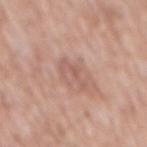No biopsy was performed on this lesion — it was imaged during a full skin examination and was not determined to be concerning. Automated image analysis of the tile measured a lesion area of about 4 mm², an outline eccentricity of about 0.9 (0 = round, 1 = elongated), and a symmetry-axis asymmetry near 0.55. Imaged with white-light lighting. Measured at roughly 3.5 mm in maximum diameter. Cropped from a whole-body photographic skin survey; the tile spans about 15 mm. From the mid back. A male subject in their mid- to late 50s.